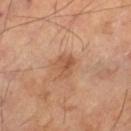Assessment:
Recorded during total-body skin imaging; not selected for excision or biopsy.
Background:
A lesion tile, about 15 mm wide, cut from a 3D total-body photograph. On the left thigh. A male subject, roughly 70 years of age.